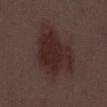Case summary:
- workup — no biopsy performed (imaged during a skin exam)
- imaging modality — ~15 mm crop, total-body skin-cancer survey
- TBP lesion metrics — an automated nevus-likeness rating near 85 out of 100
- illumination — white-light
- anatomic site — the chest
- subject — male, aged 53 to 57
- diameter — ≈7.5 mm